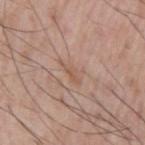follow-up: no biopsy performed (imaged during a skin exam)
body site: the left upper arm
lesion diameter: ≈3 mm
subject: male, aged around 55
image-analysis metrics: a footprint of about 4 mm², an eccentricity of roughly 0.85, and a shape-asymmetry score of about 0.4 (0 = symmetric); a lesion–skin lightness drop of about 6 and a normalized border contrast of about 4.5
image: ~15 mm tile from a whole-body skin photo